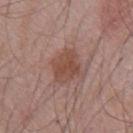Captured during whole-body skin photography for melanoma surveillance; the lesion was not biopsied. A male subject, aged 68–72. A roughly 15 mm field-of-view crop from a total-body skin photograph. The lesion is on the chest.